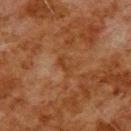A male patient, aged 78 to 82. The total-body-photography lesion software estimated an area of roughly 3.5 mm², a shape eccentricity near 0.85, and two-axis asymmetry of about 0.25. The analysis additionally found an average lesion color of about L≈31 a*≈19 b*≈30 (CIELAB), about 5 CIELAB-L* units darker than the surrounding skin, and a normalized lesion–skin contrast near 5. And it measured a classifier nevus-likeness of about 0/100 and a detector confidence of about 100 out of 100 that the crop contains a lesion. A lesion tile, about 15 mm wide, cut from a 3D total-body photograph. The lesion is located on the upper back.